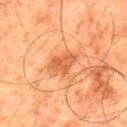follow-up: no biopsy performed (imaged during a skin exam)
body site: the left thigh
image source: 15 mm crop, total-body photography
illumination: cross-polarized illumination
lesion diameter: ~3 mm (longest diameter)
subject: male, in their 80s